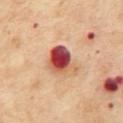{
  "biopsy_status": "not biopsied; imaged during a skin examination",
  "lighting": "cross-polarized",
  "image": {
    "source": "total-body photography crop",
    "field_of_view_mm": 15
  },
  "site": "mid back",
  "patient": {
    "sex": "female",
    "age_approx": 55
  }
}